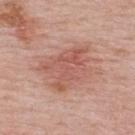Q: Was a biopsy performed?
A: total-body-photography surveillance lesion; no biopsy
Q: What kind of image is this?
A: 15 mm crop, total-body photography
Q: Where on the body is the lesion?
A: the upper back
Q: How was the tile lit?
A: white-light
Q: Who is the patient?
A: female, roughly 50 years of age